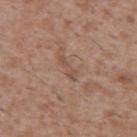This lesion was catalogued during total-body skin photography and was not selected for biopsy.
Automated tile analysis of the lesion measured a mean CIELAB color near L≈50 a*≈19 b*≈28 and a normalized lesion–skin contrast near 5.5. The analysis additionally found peripheral color asymmetry of about 0. And it measured lesion-presence confidence of about 95/100.
From the upper back.
A 15 mm close-up extracted from a 3D total-body photography capture.
The subject is a male approximately 45 years of age.
Captured under white-light illumination.
Approximately 3 mm at its widest.